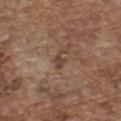acquisition: ~15 mm tile from a whole-body skin photo
illumination: white-light
body site: the chest
diameter: about 2.5 mm
patient: male, about 75 years old